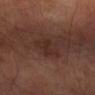Assessment: Imaged during a routine full-body skin examination; the lesion was not biopsied and no histopathology is available. Background: A lesion tile, about 15 mm wide, cut from a 3D total-body photograph. The lesion is located on the right forearm. A subject in their mid-50s.